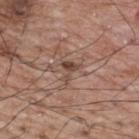biopsy_status: not biopsied; imaged during a skin examination
lighting: white-light
patient:
  sex: male
  age_approx: 70
site: upper back
image:
  source: total-body photography crop
  field_of_view_mm: 15
lesion_size:
  long_diameter_mm_approx: 3.0